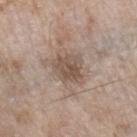Findings:
- notes — total-body-photography surveillance lesion; no biopsy
- TBP lesion metrics — about 10 CIELAB-L* units darker than the surrounding skin; radial color variation of about 1.5
- anatomic site — the left forearm
- subject — male, aged 58–62
- imaging modality — ~15 mm crop, total-body skin-cancer survey
- illumination — white-light